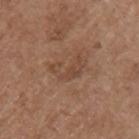Clinical impression:
Recorded during total-body skin imaging; not selected for excision or biopsy.
Context:
A female subject aged 63 to 67. Automated tile analysis of the lesion measured an average lesion color of about L≈45 a*≈19 b*≈29 (CIELAB), about 7 CIELAB-L* units darker than the surrounding skin, and a lesion-to-skin contrast of about 5.5 (normalized; higher = more distinct). And it measured a nevus-likeness score of about 0/100. A 15 mm close-up tile from a total-body photography series done for melanoma screening. On the left upper arm. Imaged with white-light lighting. The lesion's longest dimension is about 4 mm.